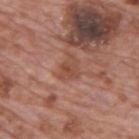workup: total-body-photography surveillance lesion; no biopsy | body site: the upper back | lesion size: ~2.5 mm (longest diameter) | illumination: white-light | patient: male, in their 70s | image source: ~15 mm crop, total-body skin-cancer survey.